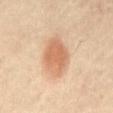The lesion was tiled from a total-body skin photograph and was not biopsied. The lesion is on the abdomen. Measured at roughly 4.5 mm in maximum diameter. A male patient, aged 63–67. A 15 mm crop from a total-body photograph taken for skin-cancer surveillance. Imaged with cross-polarized lighting.